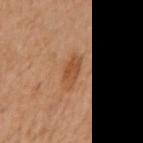Background:
From the left arm. About 3.5 mm across. A female patient, aged approximately 60. This image is a 15 mm lesion crop taken from a total-body photograph. Automated image analysis of the tile measured a lesion color around L≈43 a*≈20 b*≈32 in CIELAB and about 8 CIELAB-L* units darker than the surrounding skin. And it measured a nevus-likeness score of about 60/100. The tile uses cross-polarized illumination.